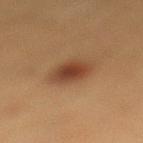<lesion>
<biopsy_status>not biopsied; imaged during a skin examination</biopsy_status>
<site>leg</site>
<image>
  <source>total-body photography crop</source>
  <field_of_view_mm>15</field_of_view_mm>
</image>
<patient>
  <sex>female</sex>
  <age_approx>40</age_approx>
</patient>
<lighting>cross-polarized</lighting>
<lesion_size>
  <long_diameter_mm_approx>3.5</long_diameter_mm_approx>
</lesion_size>
<automated_metrics>
  <lesion_detection_confidence_0_100>100</lesion_detection_confidence_0_100>
</automated_metrics>
</lesion>